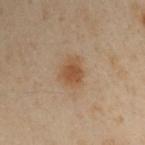Captured during whole-body skin photography for melanoma surveillance; the lesion was not biopsied. The tile uses cross-polarized illumination. Cropped from a total-body skin-imaging series; the visible field is about 15 mm. The lesion is located on the left arm. A male patient, about 50 years old. Automated tile analysis of the lesion measured a lesion area of about 5.5 mm², a shape eccentricity near 0.5, and a symmetry-axis asymmetry near 0.25. The analysis additionally found a mean CIELAB color near L≈40 a*≈15 b*≈28, a lesion–skin lightness drop of about 8, and a normalized lesion–skin contrast near 8.